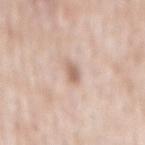Findings:
• biopsy status · catalogued during a skin exam; not biopsied
• patient · male, aged around 50
• image · ~15 mm crop, total-body skin-cancer survey
• tile lighting · white-light
• site · the mid back
• lesion diameter · ~2.5 mm (longest diameter)